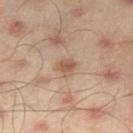No biopsy was performed on this lesion — it was imaged during a full skin examination and was not determined to be concerning. Automated tile analysis of the lesion measured an average lesion color of about L≈55 a*≈19 b*≈29 (CIELAB) and about 9 CIELAB-L* units darker than the surrounding skin. It also reported an automated nevus-likeness rating near 10 out of 100 and a detector confidence of about 100 out of 100 that the crop contains a lesion. The subject is a male in their mid-40s. The recorded lesion diameter is about 2.5 mm. From the right thigh. This is a cross-polarized tile. A 15 mm crop from a total-body photograph taken for skin-cancer surveillance.